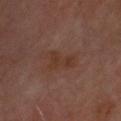Case summary:
* follow-up — total-body-photography surveillance lesion; no biopsy
* subject — in their mid- to late 60s
* body site — the head or neck
* image source — ~15 mm crop, total-body skin-cancer survey
* lesion size — ≈4.5 mm